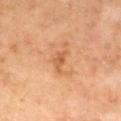| key | value |
|---|---|
| notes | total-body-photography surveillance lesion; no biopsy |
| patient | male, aged 53 to 57 |
| lighting | cross-polarized illumination |
| body site | the mid back |
| image | ~15 mm crop, total-body skin-cancer survey |